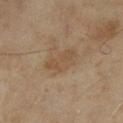Q: What did automated image analysis measure?
A: a lesion-to-skin contrast of about 5.5 (normalized; higher = more distinct); a classifier nevus-likeness of about 0/100 and lesion-presence confidence of about 100/100
Q: What is the lesion's diameter?
A: ~4 mm (longest diameter)
Q: Lesion location?
A: the left lower leg
Q: Who is the patient?
A: female, aged approximately 60
Q: What kind of image is this?
A: 15 mm crop, total-body photography